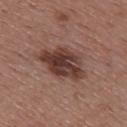Notes:
- biopsy status — no biopsy performed (imaged during a skin exam)
- acquisition — total-body-photography crop, ~15 mm field of view
- lesion diameter — ≈6 mm
- illumination — white-light
- body site — the upper back
- image-analysis metrics — a mean CIELAB color near L≈38 a*≈20 b*≈23; an automated nevus-likeness rating near 85 out of 100
- patient — female, roughly 50 years of age